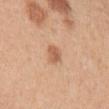The lesion is located on the left upper arm. A 15 mm close-up extracted from a 3D total-body photography capture. Automated image analysis of the tile measured a lesion area of about 5 mm² and an outline eccentricity of about 0.6 (0 = round, 1 = elongated). The software also gave an average lesion color of about L≈61 a*≈22 b*≈34 (CIELAB), about 9 CIELAB-L* units darker than the surrounding skin, and a normalized border contrast of about 6. The analysis additionally found a border-irregularity index near 2/10 and radial color variation of about 1. The patient is a female in their mid-40s. Captured under white-light illumination. Measured at roughly 3 mm in maximum diameter.